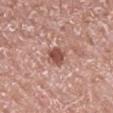{"patient": {"sex": "male", "age_approx": 70}, "image": {"source": "total-body photography crop", "field_of_view_mm": 15}, "site": "mid back", "lighting": "white-light", "automated_metrics": {"area_mm2_approx": 5.0, "eccentricity": 0.65}, "lesion_size": {"long_diameter_mm_approx": 2.5}}